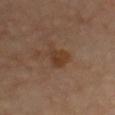Q: Was a biopsy performed?
A: total-body-photography surveillance lesion; no biopsy
Q: What did automated image analysis measure?
A: an average lesion color of about L≈37 a*≈18 b*≈30 (CIELAB), roughly 7 lightness units darker than nearby skin, and a normalized lesion–skin contrast near 7.5
Q: Where on the body is the lesion?
A: the chest
Q: Who is the patient?
A: male, aged 63–67
Q: What is the imaging modality?
A: 15 mm crop, total-body photography
Q: What lighting was used for the tile?
A: cross-polarized illumination
Q: How large is the lesion?
A: ≈3.5 mm